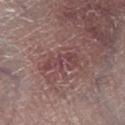biopsy status: catalogued during a skin exam; not biopsied
TBP lesion metrics: an area of roughly 7.5 mm², a shape eccentricity near 0.9, and a shape-asymmetry score of about 0.4 (0 = symmetric); an automated nevus-likeness rating near 0 out of 100 and a detector confidence of about 100 out of 100 that the crop contains a lesion
imaging modality: 15 mm crop, total-body photography
tile lighting: white-light
patient: male, aged 53 to 57
diameter: ~4.5 mm (longest diameter)
body site: the leg